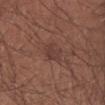workup: total-body-photography surveillance lesion; no biopsy
patient: male, aged 28–32
body site: the right forearm
image: ~15 mm tile from a whole-body skin photo
lesion diameter: ≈3 mm
tile lighting: white-light